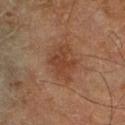<lesion>
<biopsy_status>not biopsied; imaged during a skin examination</biopsy_status>
<patient>
  <sex>male</sex>
  <age_approx>70</age_approx>
</patient>
<lighting>cross-polarized</lighting>
<image>
  <source>total-body photography crop</source>
  <field_of_view_mm>15</field_of_view_mm>
</image>
<lesion_size>
  <long_diameter_mm_approx>4.5</long_diameter_mm_approx>
</lesion_size>
<site>right lower leg</site>
</lesion>